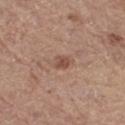biopsy_status: not biopsied; imaged during a skin examination
image:
  source: total-body photography crop
  field_of_view_mm: 15
site: right thigh
patient:
  sex: male
  age_approx: 65
lighting: white-light
automated_metrics:
  area_mm2_approx: 3.5
  border_irregularity_0_10: 2.5
  color_variation_0_10: 1.5
  peripheral_color_asymmetry: 0.5
  nevus_likeness_0_100: 50
  lesion_detection_confidence_0_100: 100
lesion_size:
  long_diameter_mm_approx: 2.5